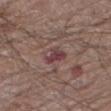<lesion>
<lesion_size>
  <long_diameter_mm_approx>3.0</long_diameter_mm_approx>
</lesion_size>
<site>leg</site>
<image>
  <source>total-body photography crop</source>
  <field_of_view_mm>15</field_of_view_mm>
</image>
<automated_metrics>
  <area_mm2_approx>4.0</area_mm2_approx>
  <eccentricity>0.75</eccentricity>
  <shape_asymmetry>0.45</shape_asymmetry>
  <cielab_L>40</cielab_L>
  <cielab_a>22</cielab_a>
  <cielab_b>16</cielab_b>
  <vs_skin_darker_L>9.0</vs_skin_darker_L>
  <vs_skin_contrast_norm>9.0</vs_skin_contrast_norm>
  <border_irregularity_0_10>4.5</border_irregularity_0_10>
  <color_variation_0_10>3.5</color_variation_0_10>
  <peripheral_color_asymmetry>1.0</peripheral_color_asymmetry>
</automated_metrics>
<patient>
  <sex>male</sex>
  <age_approx>65</age_approx>
</patient>
</lesion>